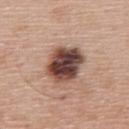Q: Is there a histopathology result?
A: total-body-photography surveillance lesion; no biopsy
Q: Patient demographics?
A: male, aged 58–62
Q: Lesion location?
A: the back
Q: What is the imaging modality?
A: total-body-photography crop, ~15 mm field of view
Q: Automated lesion metrics?
A: a lesion area of about 16 mm² and a symmetry-axis asymmetry near 0.15; a mean CIELAB color near L≈45 a*≈20 b*≈23, roughly 21 lightness units darker than nearby skin, and a normalized lesion–skin contrast near 14.5; border irregularity of about 1.5 on a 0–10 scale and internal color variation of about 9 on a 0–10 scale; a detector confidence of about 100 out of 100 that the crop contains a lesion
Q: How large is the lesion?
A: ≈5 mm